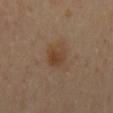Q: Was this lesion biopsied?
A: imaged on a skin check; not biopsied
Q: What kind of image is this?
A: 15 mm crop, total-body photography
Q: Illumination type?
A: cross-polarized illumination
Q: Who is the patient?
A: female, about 55 years old
Q: How large is the lesion?
A: ~3.5 mm (longest diameter)
Q: What is the anatomic site?
A: the mid back